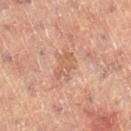{"biopsy_status": "not biopsied; imaged during a skin examination", "image": {"source": "total-body photography crop", "field_of_view_mm": 15}, "automated_metrics": {"area_mm2_approx": 5.5, "eccentricity": 0.8, "shape_asymmetry": 0.25, "border_irregularity_0_10": 3.5, "color_variation_0_10": 2.5}, "lighting": "cross-polarized", "lesion_size": {"long_diameter_mm_approx": 3.0}, "site": "left leg", "patient": {"sex": "female", "age_approx": 80}}